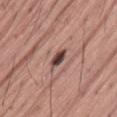This lesion was catalogued during total-body skin photography and was not selected for biopsy. The total-body-photography lesion software estimated a lesion area of about 3.5 mm², an outline eccentricity of about 0.8 (0 = round, 1 = elongated), and a symmetry-axis asymmetry near 0.2. It also reported border irregularity of about 1.5 on a 0–10 scale, a color-variation rating of about 4.5/10, and peripheral color asymmetry of about 1.5. The analysis additionally found a classifier nevus-likeness of about 25/100 and a detector confidence of about 100 out of 100 that the crop contains a lesion. A roughly 15 mm field-of-view crop from a total-body skin photograph. The lesion is on the lower back. Imaged with white-light lighting. Longest diameter approximately 2.5 mm. A male patient, aged around 45.